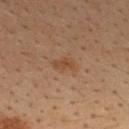| feature | finding |
|---|---|
| follow-up | total-body-photography surveillance lesion; no biopsy |
| diameter | about 2.5 mm |
| illumination | cross-polarized illumination |
| image | 15 mm crop, total-body photography |
| subject | male, aged around 35 |
| site | the upper back |